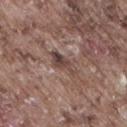Q: Is there a histopathology result?
A: catalogued during a skin exam; not biopsied
Q: What kind of image is this?
A: total-body-photography crop, ~15 mm field of view
Q: Patient demographics?
A: male, about 75 years old
Q: What is the anatomic site?
A: the right thigh
Q: How was the tile lit?
A: white-light illumination
Q: Automated lesion metrics?
A: an average lesion color of about L≈43 a*≈16 b*≈20 (CIELAB) and roughly 10 lightness units darker than nearby skin; border irregularity of about 3.5 on a 0–10 scale and a color-variation rating of about 5.5/10; lesion-presence confidence of about 85/100